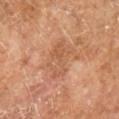notes=total-body-photography surveillance lesion; no biopsy
image-analysis metrics=an eccentricity of roughly 0.7 and two-axis asymmetry of about 0.3; a normalized border contrast of about 4.5
lighting=cross-polarized illumination
image source=~15 mm tile from a whole-body skin photo
subject=male, about 65 years old
lesion diameter=≈5 mm